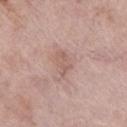follow-up: catalogued during a skin exam; not biopsied | lighting: white-light illumination | imaging modality: ~15 mm tile from a whole-body skin photo | subject: female, approximately 75 years of age | lesion diameter: ≈3.5 mm | location: the right lower leg.